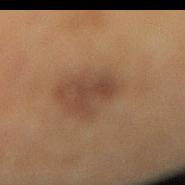follow-up: total-body-photography surveillance lesion; no biopsy | size: about 3 mm | subject: female, aged 38 to 42 | image: ~15 mm tile from a whole-body skin photo | illumination: cross-polarized illumination | location: the leg.